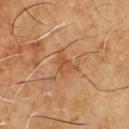Clinical impression: This lesion was catalogued during total-body skin photography and was not selected for biopsy. Clinical summary: A lesion tile, about 15 mm wide, cut from a 3D total-body photograph. Located on the front of the torso. This is a cross-polarized tile. The total-body-photography lesion software estimated an outline eccentricity of about 0.7 (0 = round, 1 = elongated) and a shape-asymmetry score of about 0.45 (0 = symmetric). The analysis additionally found lesion-presence confidence of about 100/100. The lesion's longest dimension is about 3.5 mm. A male patient approximately 60 years of age.